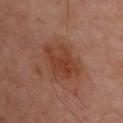Assessment: Captured during whole-body skin photography for melanoma surveillance; the lesion was not biopsied. Background: Imaged with cross-polarized lighting. Located on the back. This image is a 15 mm lesion crop taken from a total-body photograph. The subject is a male roughly 50 years of age.